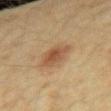Notes:
* follow-up · catalogued during a skin exam; not biopsied
* location · the right forearm
* lesion diameter · ≈3 mm
* acquisition · total-body-photography crop, ~15 mm field of view
* patient · female, aged approximately 40
* automated lesion analysis · a footprint of about 6.5 mm², an eccentricity of roughly 0.35, and a shape-asymmetry score of about 0.2 (0 = symmetric); an average lesion color of about L≈43 a*≈17 b*≈29 (CIELAB) and a lesion-to-skin contrast of about 7.5 (normalized; higher = more distinct); a border-irregularity index near 2.5/10 and internal color variation of about 3.5 on a 0–10 scale